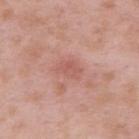workup = no biopsy performed (imaged during a skin exam); lesion size = ≈3 mm; imaging modality = ~15 mm crop, total-body skin-cancer survey; tile lighting = white-light illumination; patient = male, approximately 55 years of age; site = the upper back.